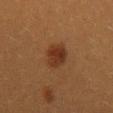The tile uses cross-polarized illumination. An algorithmic analysis of the crop reported a mean CIELAB color near L≈27 a*≈19 b*≈27 and about 8 CIELAB-L* units darker than the surrounding skin. It also reported a border-irregularity rating of about 2/10, a color-variation rating of about 3/10, and a peripheral color-asymmetry measure near 1. The analysis additionally found a classifier nevus-likeness of about 95/100 and a lesion-detection confidence of about 100/100. The subject is a female in their 40s. From the mid back. About 3.5 mm across. A 15 mm crop from a total-body photograph taken for skin-cancer surveillance.